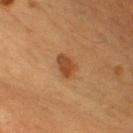Findings:
- lesion size — about 3.5 mm
- imaging modality — ~15 mm tile from a whole-body skin photo
- location — the front of the torso
- subject — male, in their 50s
- illumination — cross-polarized illumination